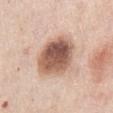The lesion was tiled from a total-body skin photograph and was not biopsied. The patient is a male approximately 75 years of age. On the front of the torso. A lesion tile, about 15 mm wide, cut from a 3D total-body photograph.